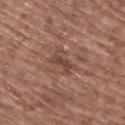<lesion>
<biopsy_status>not biopsied; imaged during a skin examination</biopsy_status>
<lesion_size>
  <long_diameter_mm_approx>3.5</long_diameter_mm_approx>
</lesion_size>
<image>
  <source>total-body photography crop</source>
  <field_of_view_mm>15</field_of_view_mm>
</image>
<site>upper back</site>
<lighting>white-light</lighting>
<patient>
  <sex>female</sex>
  <age_approx>75</age_approx>
</patient>
</lesion>